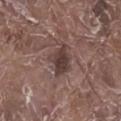No biopsy was performed on this lesion — it was imaged during a full skin examination and was not determined to be concerning.
Located on the right lower leg.
The patient is a male aged approximately 80.
A 15 mm crop from a total-body photograph taken for skin-cancer surveillance.